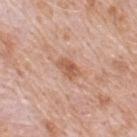biopsy_status: not biopsied; imaged during a skin examination
lesion_size:
  long_diameter_mm_approx: 3.0
image:
  source: total-body photography crop
  field_of_view_mm: 15
lighting: white-light
patient:
  sex: male
  age_approx: 50
site: upper back
automated_metrics:
  area_mm2_approx: 4.0
  eccentricity: 0.8
  shape_asymmetry: 0.25
  cielab_L: 57
  cielab_a: 23
  cielab_b: 32
  vs_skin_darker_L: 11.0
  vs_skin_contrast_norm: 7.5